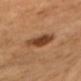No biopsy was performed on this lesion — it was imaged during a full skin examination and was not determined to be concerning. Automated tile analysis of the lesion measured a nevus-likeness score of about 90/100 and a lesion-detection confidence of about 100/100. About 4 mm across. A female subject roughly 70 years of age. A 15 mm crop from a total-body photograph taken for skin-cancer surveillance. The tile uses cross-polarized illumination. The lesion is located on the head or neck.